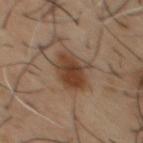The lesion was tiled from a total-body skin photograph and was not biopsied.
Cropped from a total-body skin-imaging series; the visible field is about 15 mm.
Captured under cross-polarized illumination.
The lesion is located on the front of the torso.
The lesion-visualizer software estimated an area of roughly 10 mm² and a shape eccentricity near 0.75. It also reported a border-irregularity rating of about 3.5/10, a color-variation rating of about 4.5/10, and radial color variation of about 1.5. And it measured lesion-presence confidence of about 100/100.
A male patient, in their mid- to late 50s.